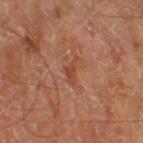Case summary:
* follow-up · no biopsy performed (imaged during a skin exam)
* diameter · ≈3 mm
* automated metrics · a border-irregularity index near 6.5/10, a color-variation rating of about 0/10, and peripheral color asymmetry of about 0; a nevus-likeness score of about 0/100 and lesion-presence confidence of about 100/100
* image source · ~15 mm crop, total-body skin-cancer survey
* body site · the right thigh
* subject · male, about 70 years old